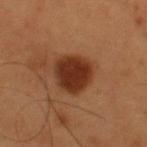Q: Who is the patient?
A: male, aged 53–57
Q: What kind of image is this?
A: total-body-photography crop, ~15 mm field of view
Q: What did automated image analysis measure?
A: a footprint of about 14 mm² and an outline eccentricity of about 0.2 (0 = round, 1 = elongated)
Q: What is the anatomic site?
A: the upper back